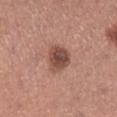No biopsy was performed on this lesion — it was imaged during a full skin examination and was not determined to be concerning. An algorithmic analysis of the crop reported a footprint of about 8 mm². The analysis additionally found a mean CIELAB color near L≈48 a*≈23 b*≈26 and a normalized border contrast of about 9. The software also gave a border-irregularity rating of about 2/10, a within-lesion color-variation index near 4.5/10, and peripheral color asymmetry of about 1.5. And it measured a classifier nevus-likeness of about 65/100. A lesion tile, about 15 mm wide, cut from a 3D total-body photograph. Imaged with white-light lighting. The lesion is located on the right lower leg. The recorded lesion diameter is about 3.5 mm. A female patient, aged 28–32.